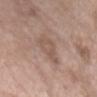<record>
  <biopsy_status>not biopsied; imaged during a skin examination</biopsy_status>
  <lighting>white-light</lighting>
  <site>chest</site>
  <patient>
    <sex>female</sex>
    <age_approx>75</age_approx>
  </patient>
  <image>
    <source>total-body photography crop</source>
    <field_of_view_mm>15</field_of_view_mm>
  </image>
</record>